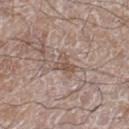notes = total-body-photography surveillance lesion; no biopsy | illumination = white-light illumination | subject = male, roughly 60 years of age | image source = total-body-photography crop, ~15 mm field of view | body site = the right lower leg | diameter = ~2.5 mm (longest diameter).